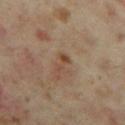No biopsy was performed on this lesion — it was imaged during a full skin examination and was not determined to be concerning.